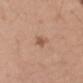| field | value |
|---|---|
| biopsy status | catalogued during a skin exam; not biopsied |